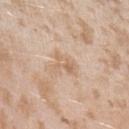Findings:
- workup — catalogued during a skin exam; not biopsied